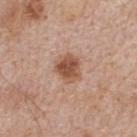An algorithmic analysis of the crop reported a border-irregularity index near 1.5/10 and a color-variation rating of about 4/10. The software also gave a detector confidence of about 100 out of 100 that the crop contains a lesion.
A male subject roughly 65 years of age.
Cropped from a whole-body photographic skin survey; the tile spans about 15 mm.
On the upper back.
Imaged with white-light lighting.